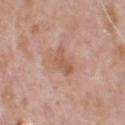Findings:
* lighting: white-light illumination
* site: the head or neck
* imaging modality: ~15 mm tile from a whole-body skin photo
* subject: male, in their 70s